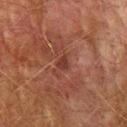Impression: This lesion was catalogued during total-body skin photography and was not selected for biopsy. Image and clinical context: The subject is a male aged around 75. About 2 mm across. The lesion-visualizer software estimated a lesion color around L≈30 a*≈22 b*≈23 in CIELAB, roughly 7 lightness units darker than nearby skin, and a lesion-to-skin contrast of about 7 (normalized; higher = more distinct). And it measured a border-irregularity index near 3/10, internal color variation of about 1 on a 0–10 scale, and peripheral color asymmetry of about 0.5. It also reported lesion-presence confidence of about 100/100. The lesion is on the left upper arm. This image is a 15 mm lesion crop taken from a total-body photograph. The tile uses cross-polarized illumination.